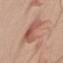{
  "biopsy_status": "not biopsied; imaged during a skin examination",
  "patient": {
    "sex": "male",
    "age_approx": 50
  },
  "image": {
    "source": "total-body photography crop",
    "field_of_view_mm": 15
  },
  "automated_metrics": {
    "area_mm2_approx": 12.0,
    "shape_asymmetry": 0.45,
    "cielab_L": 55,
    "cielab_a": 24,
    "cielab_b": 28,
    "vs_skin_darker_L": 10.0,
    "vs_skin_contrast_norm": 7.0
  },
  "site": "arm"
}